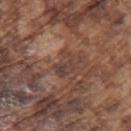Notes:
- lighting — white-light
- lesion diameter — ≈3 mm
- image-analysis metrics — a footprint of about 5 mm² and an outline eccentricity of about 0.75 (0 = round, 1 = elongated); an average lesion color of about L≈40 a*≈18 b*≈23 (CIELAB) and about 8 CIELAB-L* units darker than the surrounding skin; radial color variation of about 1; a nevus-likeness score of about 0/100
- imaging modality — 15 mm crop, total-body photography
- location — the left upper arm
- patient — male, approximately 75 years of age